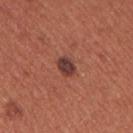notes=total-body-photography surveillance lesion; no biopsy | image=~15 mm tile from a whole-body skin photo | body site=the arm | subject=female, approximately 35 years of age | tile lighting=white-light illumination | size=~2.5 mm (longest diameter) | automated metrics=an area of roughly 4 mm² and an eccentricity of roughly 0.7; a mean CIELAB color near L≈38 a*≈24 b*≈23 and a normalized border contrast of about 11; an automated nevus-likeness rating near 100 out of 100.